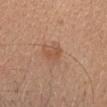workup: total-body-photography surveillance lesion; no biopsy | acquisition: 15 mm crop, total-body photography | TBP lesion metrics: a border-irregularity index near 3/10, internal color variation of about 1 on a 0–10 scale, and peripheral color asymmetry of about 0.5; a classifier nevus-likeness of about 35/100 and lesion-presence confidence of about 100/100 | patient: female, aged approximately 40 | anatomic site: the head or neck | tile lighting: white-light illumination.